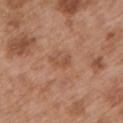Part of a total-body skin-imaging series; this lesion was reviewed on a skin check and was not flagged for biopsy. A 15 mm close-up extracted from a 3D total-body photography capture. Longest diameter approximately 2.5 mm. Captured under white-light illumination. The lesion is located on the arm. The patient is a male approximately 55 years of age.